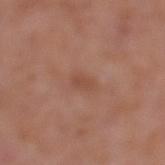This lesion was catalogued during total-body skin photography and was not selected for biopsy. A roughly 15 mm field-of-view crop from a total-body skin photograph. The recorded lesion diameter is about 2.5 mm. The lesion is on the left lower leg. This is a white-light tile. The subject is a female about 40 years old. Automated image analysis of the tile measured a footprint of about 2.5 mm² and a shape-asymmetry score of about 0.2 (0 = symmetric). The software also gave a classifier nevus-likeness of about 0/100 and a lesion-detection confidence of about 100/100.